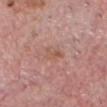notes: no biopsy performed (imaged during a skin exam) | size: ≈3 mm | location: the head or neck | subject: male, about 60 years old | imaging modality: ~15 mm tile from a whole-body skin photo.